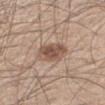- notes · total-body-photography surveillance lesion; no biopsy